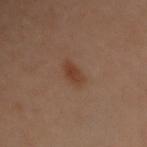The lesion was photographed on a routine skin check and not biopsied; there is no pathology result. Located on the back. Captured under cross-polarized illumination. Automated image analysis of the tile measured a mean CIELAB color near L≈33 a*≈18 b*≈26, about 7 CIELAB-L* units darker than the surrounding skin, and a lesion-to-skin contrast of about 7.5 (normalized; higher = more distinct). A female patient, aged around 60. A close-up tile cropped from a whole-body skin photograph, about 15 mm across.